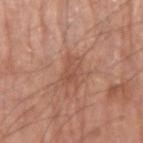{"biopsy_status": "not biopsied; imaged during a skin examination", "lighting": "white-light", "site": "right upper arm", "automated_metrics": {"area_mm2_approx": 4.5, "eccentricity": 0.9, "shape_asymmetry": 0.25}, "patient": {"sex": "male", "age_approx": 75}, "image": {"source": "total-body photography crop", "field_of_view_mm": 15}, "lesion_size": {"long_diameter_mm_approx": 3.5}}